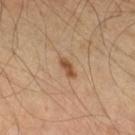<case>
  <biopsy_status>not biopsied; imaged during a skin examination</biopsy_status>
  <lesion_size>
    <long_diameter_mm_approx>2.5</long_diameter_mm_approx>
  </lesion_size>
  <patient>
    <sex>male</sex>
    <age_approx>45</age_approx>
  </patient>
  <lighting>cross-polarized</lighting>
  <site>left forearm</site>
  <image>
    <source>total-body photography crop</source>
    <field_of_view_mm>15</field_of_view_mm>
  </image>
</case>